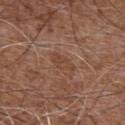The lesion is on the abdomen. The tile uses white-light illumination. The patient is a male aged 73–77. A 15 mm close-up extracted from a 3D total-body photography capture. The total-body-photography lesion software estimated a footprint of about 4 mm², an eccentricity of roughly 0.9, and a shape-asymmetry score of about 0.35 (0 = symmetric). The software also gave an average lesion color of about L≈43 a*≈21 b*≈29 (CIELAB), a lesion–skin lightness drop of about 6, and a lesion-to-skin contrast of about 5 (normalized; higher = more distinct). It also reported a border-irregularity index near 4/10 and radial color variation of about 0.5. The analysis additionally found an automated nevus-likeness rating near 0 out of 100 and a lesion-detection confidence of about 100/100. Measured at roughly 3 mm in maximum diameter.